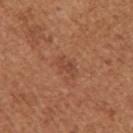The lesion-visualizer software estimated about 7 CIELAB-L* units darker than the surrounding skin. The software also gave a border-irregularity index near 3.5/10, a color-variation rating of about 1.5/10, and a peripheral color-asymmetry measure near 0.5. It also reported a nevus-likeness score of about 5/100 and a detector confidence of about 100 out of 100 that the crop contains a lesion. This is a white-light tile. On the right upper arm. Cropped from a whole-body photographic skin survey; the tile spans about 15 mm. A female subject in their 40s. The lesion's longest dimension is about 3 mm.